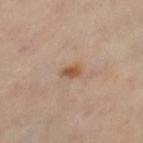Captured during whole-body skin photography for melanoma surveillance; the lesion was not biopsied.
The tile uses cross-polarized illumination.
From the leg.
The subject is a female about 45 years old.
A 15 mm close-up tile from a total-body photography series done for melanoma screening.
An algorithmic analysis of the crop reported a footprint of about 3 mm², a shape eccentricity near 0.75, and two-axis asymmetry of about 0.2. And it measured a border-irregularity index near 1.5/10 and peripheral color asymmetry of about 1. It also reported a classifier nevus-likeness of about 85/100 and lesion-presence confidence of about 100/100.